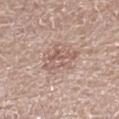No biopsy was performed on this lesion — it was imaged during a full skin examination and was not determined to be concerning. Located on the right lower leg. A female subject aged 68 to 72. A roughly 15 mm field-of-view crop from a total-body skin photograph.